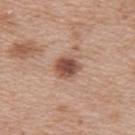Impression: The lesion was photographed on a routine skin check and not biopsied; there is no pathology result. Image and clinical context: The tile uses white-light illumination. A roughly 15 mm field-of-view crop from a total-body skin photograph. The subject is a female approximately 40 years of age. Measured at roughly 3 mm in maximum diameter. The lesion is located on the upper back. The total-body-photography lesion software estimated roughly 14 lightness units darker than nearby skin and a lesion-to-skin contrast of about 10 (normalized; higher = more distinct). It also reported internal color variation of about 4.5 on a 0–10 scale.